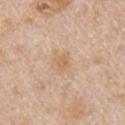The lesion was tiled from a total-body skin photograph and was not biopsied. A male patient approximately 65 years of age. Longest diameter approximately 2.5 mm. Cropped from a total-body skin-imaging series; the visible field is about 15 mm. Automated tile analysis of the lesion measured an area of roughly 3.5 mm², a shape eccentricity near 0.6, and a shape-asymmetry score of about 0.3 (0 = symmetric). The analysis additionally found a mean CIELAB color near L≈64 a*≈17 b*≈34 and a normalized border contrast of about 5.5. And it measured a classifier nevus-likeness of about 0/100 and a lesion-detection confidence of about 100/100. The lesion is located on the right upper arm. This is a white-light tile.